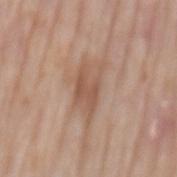{"biopsy_status": "not biopsied; imaged during a skin examination", "automated_metrics": {"area_mm2_approx": 10.0, "eccentricity": 0.9, "shape_asymmetry": 0.3, "cielab_L": 55, "cielab_a": 19, "cielab_b": 29, "vs_skin_contrast_norm": 6.5, "border_irregularity_0_10": 5.0, "color_variation_0_10": 3.5, "peripheral_color_asymmetry": 1.0, "nevus_likeness_0_100": 5, "lesion_detection_confidence_0_100": 100}, "patient": {"sex": "male", "age_approx": 75}, "lighting": "white-light", "site": "mid back", "image": {"source": "total-body photography crop", "field_of_view_mm": 15}}